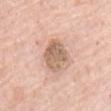Notes:
- notes: catalogued during a skin exam; not biopsied
- location: the back
- lesion diameter: about 4 mm
- lighting: white-light illumination
- image source: ~15 mm crop, total-body skin-cancer survey
- patient: male, in their 80s